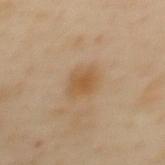| feature | finding |
|---|---|
| workup | imaged on a skin check; not biopsied |
| lighting | cross-polarized |
| subject | female, aged approximately 60 |
| lesion diameter | about 3 mm |
| automated metrics | a lesion area of about 5.5 mm², an eccentricity of roughly 0.65, and two-axis asymmetry of about 0.25; a lesion color around L≈46 a*≈15 b*≈32 in CIELAB and about 7 CIELAB-L* units darker than the surrounding skin; a classifier nevus-likeness of about 65/100 |
| acquisition | 15 mm crop, total-body photography |
| site | the upper back |